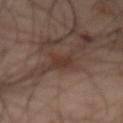The recorded lesion diameter is about 4 mm. A male patient, in their mid-60s. From the abdomen. Captured under cross-polarized illumination. A lesion tile, about 15 mm wide, cut from a 3D total-body photograph. The lesion-visualizer software estimated an area of roughly 5 mm², an eccentricity of roughly 0.9, and a shape-asymmetry score of about 0.35 (0 = symmetric). The software also gave a lesion color around L≈33 a*≈17 b*≈23 in CIELAB, about 8 CIELAB-L* units darker than the surrounding skin, and a normalized lesion–skin contrast near 8. It also reported a border-irregularity index near 4.5/10, a color-variation rating of about 1.5/10, and radial color variation of about 0.5. The analysis additionally found an automated nevus-likeness rating near 15 out of 100 and a detector confidence of about 100 out of 100 that the crop contains a lesion.